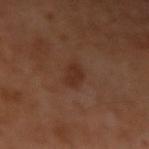The lesion was tiled from a total-body skin photograph and was not biopsied.
A male patient aged around 55.
This image is a 15 mm lesion crop taken from a total-body photograph.
An algorithmic analysis of the crop reported a footprint of about 4.5 mm², a shape eccentricity near 0.8, and a symmetry-axis asymmetry near 0.3. The software also gave a border-irregularity rating of about 3/10, internal color variation of about 1.5 on a 0–10 scale, and radial color variation of about 0.5.
The lesion is located on the left upper arm.
This is a cross-polarized tile.
Longest diameter approximately 3 mm.